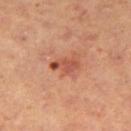Q: Was a biopsy performed?
A: total-body-photography surveillance lesion; no biopsy
Q: Illumination type?
A: cross-polarized
Q: What are the patient's age and sex?
A: female, in their 40s
Q: Lesion location?
A: the right thigh
Q: What is the imaging modality?
A: ~15 mm crop, total-body skin-cancer survey